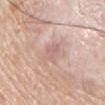Impression:
Imaged during a routine full-body skin examination; the lesion was not biopsied and no histopathology is available.
Image and clinical context:
The lesion is on the arm. This image is a 15 mm lesion crop taken from a total-body photograph. A male patient, aged around 60. Longest diameter approximately 3 mm.